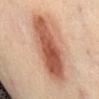Impression: Imaged during a routine full-body skin examination; the lesion was not biopsied and no histopathology is available. Context: The patient is a female aged around 40. Cropped from a whole-body photographic skin survey; the tile spans about 15 mm. From the front of the torso.